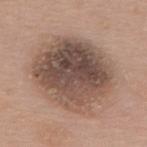No biopsy was performed on this lesion — it was imaged during a full skin examination and was not determined to be concerning.
A female patient roughly 60 years of age.
The recorded lesion diameter is about 9 mm.
On the back.
Captured under white-light illumination.
A region of skin cropped from a whole-body photographic capture, roughly 15 mm wide.